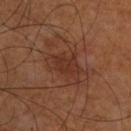Impression:
No biopsy was performed on this lesion — it was imaged during a full skin examination and was not determined to be concerning.
Image and clinical context:
From the right lower leg. The total-body-photography lesion software estimated a nevus-likeness score of about 0/100 and a detector confidence of about 100 out of 100 that the crop contains a lesion. A region of skin cropped from a whole-body photographic capture, roughly 15 mm wide. This is a cross-polarized tile. Longest diameter approximately 5 mm. A male subject, about 70 years old.